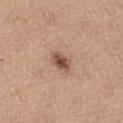Q: Was this lesion biopsied?
A: imaged on a skin check; not biopsied
Q: What did automated image analysis measure?
A: a lesion area of about 4.5 mm²; a lesion color around L≈51 a*≈21 b*≈30 in CIELAB, about 13 CIELAB-L* units darker than the surrounding skin, and a normalized lesion–skin contrast near 9; internal color variation of about 3 on a 0–10 scale and a peripheral color-asymmetry measure near 1; a classifier nevus-likeness of about 95/100 and a detector confidence of about 100 out of 100 that the crop contains a lesion
Q: What is the imaging modality?
A: ~15 mm tile from a whole-body skin photo
Q: Lesion location?
A: the leg
Q: Who is the patient?
A: female, roughly 65 years of age
Q: What lighting was used for the tile?
A: white-light illumination